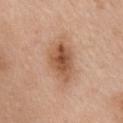Part of a total-body skin-imaging series; this lesion was reviewed on a skin check and was not flagged for biopsy. From the chest. Cropped from a total-body skin-imaging series; the visible field is about 15 mm. Captured under white-light illumination. The patient is a female about 65 years old. An algorithmic analysis of the crop reported a shape eccentricity near 0.8 and two-axis asymmetry of about 0.25. And it measured a lesion color around L≈55 a*≈21 b*≈33 in CIELAB, roughly 12 lightness units darker than nearby skin, and a normalized lesion–skin contrast near 8. It also reported peripheral color asymmetry of about 2. The software also gave a nevus-likeness score of about 90/100 and a detector confidence of about 100 out of 100 that the crop contains a lesion. Longest diameter approximately 6 mm.